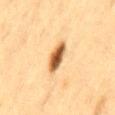| field | value |
|---|---|
| biopsy status | catalogued during a skin exam; not biopsied |
| lighting | cross-polarized |
| image source | ~15 mm tile from a whole-body skin photo |
| lesion diameter | about 4 mm |
| location | the lower back |
| patient | female, aged approximately 40 |